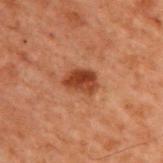A male patient, approximately 70 years of age. Cropped from a whole-body photographic skin survey; the tile spans about 15 mm. Located on the upper back.